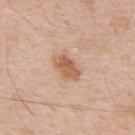Impression:
Imaged during a routine full-body skin examination; the lesion was not biopsied and no histopathology is available.
Clinical summary:
A region of skin cropped from a whole-body photographic capture, roughly 15 mm wide. A male subject, aged around 50. An algorithmic analysis of the crop reported a mean CIELAB color near L≈60 a*≈20 b*≈33 and a lesion–skin lightness drop of about 11. And it measured a border-irregularity rating of about 2/10, a within-lesion color-variation index near 3.5/10, and radial color variation of about 1. And it measured a nevus-likeness score of about 50/100 and lesion-presence confidence of about 100/100. Captured under white-light illumination. On the upper back.